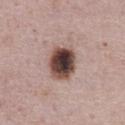image source: 15 mm crop, total-body photography | patient: male, approximately 75 years of age | anatomic site: the abdomen | tile lighting: white-light illumination.